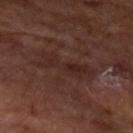This lesion was catalogued during total-body skin photography and was not selected for biopsy.